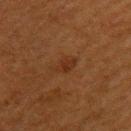Captured during whole-body skin photography for melanoma surveillance; the lesion was not biopsied.
Imaged with cross-polarized lighting.
From the upper back.
A 15 mm crop from a total-body photograph taken for skin-cancer surveillance.
Automated image analysis of the tile measured a lesion color around L≈26 a*≈19 b*≈28 in CIELAB, about 5 CIELAB-L* units darker than the surrounding skin, and a normalized border contrast of about 5.5. The software also gave a within-lesion color-variation index near 2/10. The analysis additionally found an automated nevus-likeness rating near 10 out of 100 and a detector confidence of about 100 out of 100 that the crop contains a lesion.
The patient is a female approximately 40 years of age.